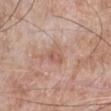Assessment:
The lesion was tiled from a total-body skin photograph and was not biopsied.
Context:
A 15 mm close-up tile from a total-body photography series done for melanoma screening. Automated image analysis of the tile measured an average lesion color of about L≈57 a*≈21 b*≈27 (CIELAB), a lesion–skin lightness drop of about 8, and a lesion-to-skin contrast of about 5.5 (normalized; higher = more distinct). The lesion is located on the right lower leg. A male patient, in their mid- to late 70s. Imaged with white-light lighting.